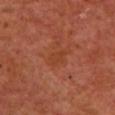The lesion was tiled from a total-body skin photograph and was not biopsied.
A female subject, approximately 55 years of age.
Imaged with cross-polarized lighting.
The recorded lesion diameter is about 2.5 mm.
Cropped from a total-body skin-imaging series; the visible field is about 15 mm.
The lesion is located on the front of the torso.